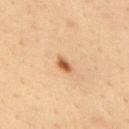Assessment: This lesion was catalogued during total-body skin photography and was not selected for biopsy. Context: The lesion is on the upper back. A close-up tile cropped from a whole-body skin photograph, about 15 mm across. A male patient, roughly 35 years of age. The recorded lesion diameter is about 2.5 mm.